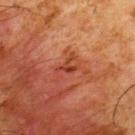| feature | finding |
|---|---|
| notes | catalogued during a skin exam; not biopsied |
| image | ~15 mm crop, total-body skin-cancer survey |
| location | the upper back |
| automated lesion analysis | an automated nevus-likeness rating near 0 out of 100 and a lesion-detection confidence of about 100/100 |
| diameter | ~3.5 mm (longest diameter) |
| subject | male, aged approximately 60 |